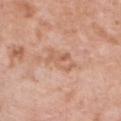biopsy status = imaged on a skin check; not biopsied | lesion diameter = ≈4 mm | image source = ~15 mm tile from a whole-body skin photo | tile lighting = white-light | patient = female, approximately 50 years of age | location = the chest.